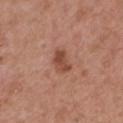Findings:
* follow-up: catalogued during a skin exam; not biopsied
* lighting: white-light illumination
* automated lesion analysis: a nevus-likeness score of about 45/100 and a detector confidence of about 100 out of 100 that the crop contains a lesion
* body site: the mid back
* subject: male, in their mid-50s
* lesion size: about 3.5 mm
* image: 15 mm crop, total-body photography